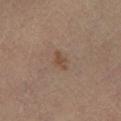Context:
The recorded lesion diameter is about 3 mm. A close-up tile cropped from a whole-body skin photograph, about 15 mm across. A female subject, approximately 55 years of age. Captured under cross-polarized illumination. The total-body-photography lesion software estimated a lesion color around L≈39 a*≈14 b*≈25 in CIELAB, roughly 6 lightness units darker than nearby skin, and a normalized lesion–skin contrast near 6.5. The analysis additionally found a border-irregularity rating of about 4.5/10, a color-variation rating of about 1/10, and a peripheral color-asymmetry measure near 0.5. It also reported an automated nevus-likeness rating near 5 out of 100 and lesion-presence confidence of about 100/100. The lesion is on the right lower leg.